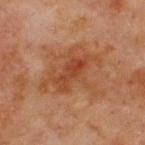Q: Was a biopsy performed?
A: imaged on a skin check; not biopsied
Q: Lesion location?
A: the chest
Q: Who is the patient?
A: male, in their mid-60s
Q: How was the tile lit?
A: cross-polarized illumination
Q: What is the imaging modality?
A: ~15 mm crop, total-body skin-cancer survey
Q: Lesion size?
A: about 7 mm